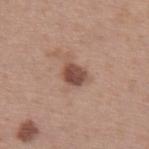Recorded during total-body skin imaging; not selected for excision or biopsy. A region of skin cropped from a whole-body photographic capture, roughly 15 mm wide. Imaged with white-light lighting. The subject is a male aged 43–47. From the left upper arm. Automated image analysis of the tile measured an automated nevus-likeness rating near 70 out of 100 and a detector confidence of about 100 out of 100 that the crop contains a lesion. Measured at roughly 3 mm in maximum diameter.